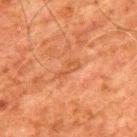Imaged during a routine full-body skin examination; the lesion was not biopsied and no histopathology is available. Located on the upper back. About 3.5 mm across. The lesion-visualizer software estimated a lesion color around L≈44 a*≈24 b*≈34 in CIELAB and a normalized border contrast of about 5. The analysis additionally found border irregularity of about 5 on a 0–10 scale, a color-variation rating of about 0.5/10, and peripheral color asymmetry of about 0. The subject is a male aged around 80. A roughly 15 mm field-of-view crop from a total-body skin photograph. Imaged with cross-polarized lighting.